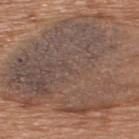No biopsy was performed on this lesion — it was imaged during a full skin examination and was not determined to be concerning. The patient is a male aged around 65. The lesion is on the upper back. A close-up tile cropped from a whole-body skin photograph, about 15 mm across. Approximately 14 mm at its widest. The tile uses white-light illumination.